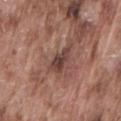The lesion was tiled from a total-body skin photograph and was not biopsied. The tile uses white-light illumination. A lesion tile, about 15 mm wide, cut from a 3D total-body photograph. The lesion-visualizer software estimated a lesion color around L≈41 a*≈20 b*≈22 in CIELAB, a lesion–skin lightness drop of about 10, and a normalized lesion–skin contrast near 8.5. The analysis additionally found a border-irregularity rating of about 4/10, a color-variation rating of about 3/10, and a peripheral color-asymmetry measure near 1. And it measured a classifier nevus-likeness of about 5/100 and a lesion-detection confidence of about 100/100. A male subject, approximately 75 years of age. Longest diameter approximately 3.5 mm. Located on the lower back.